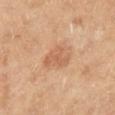Case summary:
- follow-up: imaged on a skin check; not biopsied
- body site: the right lower leg
- diameter: ≈3.5 mm
- illumination: cross-polarized
- subject: female, about 60 years old
- imaging modality: 15 mm crop, total-body photography